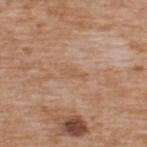Assessment: Recorded during total-body skin imaging; not selected for excision or biopsy. Background: On the upper back. The lesion-visualizer software estimated a border-irregularity rating of about 3/10 and peripheral color asymmetry of about 0. The software also gave a nevus-likeness score of about 0/100 and a lesion-detection confidence of about 100/100. A male patient, about 65 years old. A roughly 15 mm field-of-view crop from a total-body skin photograph. The tile uses white-light illumination.